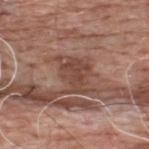This lesion was catalogued during total-body skin photography and was not selected for biopsy.
Longest diameter approximately 4.5 mm.
Located on the back.
Imaged with white-light lighting.
A male subject roughly 60 years of age.
Cropped from a whole-body photographic skin survey; the tile spans about 15 mm.